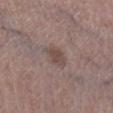notes: no biopsy performed (imaged during a skin exam) | TBP lesion metrics: an area of roughly 4.5 mm² and a shape eccentricity near 0.65; about 8 CIELAB-L* units darker than the surrounding skin and a lesion-to-skin contrast of about 6.5 (normalized; higher = more distinct) | patient: female, aged around 65 | lighting: white-light illumination | location: the left lower leg | diameter: ~2.5 mm (longest diameter) | image: ~15 mm crop, total-body skin-cancer survey.